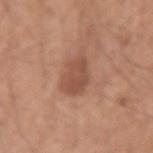{
  "biopsy_status": "not biopsied; imaged during a skin examination",
  "patient": {
    "sex": "male",
    "age_approx": 55
  },
  "lighting": "white-light",
  "lesion_size": {
    "long_diameter_mm_approx": 4.0
  },
  "image": {
    "source": "total-body photography crop",
    "field_of_view_mm": 15
  },
  "site": "left upper arm",
  "automated_metrics": {
    "cielab_L": 50,
    "cielab_a": 22,
    "cielab_b": 29,
    "border_irregularity_0_10": 2.5,
    "color_variation_0_10": 2.0,
    "peripheral_color_asymmetry": 0.5,
    "nevus_likeness_0_100": 25,
    "lesion_detection_confidence_0_100": 100
  }
}